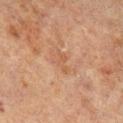Assessment: The lesion was photographed on a routine skin check and not biopsied; there is no pathology result. Background: The total-body-photography lesion software estimated an outline eccentricity of about 0.75 (0 = round, 1 = elongated) and a symmetry-axis asymmetry near 0.45. It also reported roughly 5 lightness units darker than nearby skin and a lesion-to-skin contrast of about 4.5 (normalized; higher = more distinct). The analysis additionally found a border-irregularity rating of about 5/10, a color-variation rating of about 2/10, and a peripheral color-asymmetry measure near 1. And it measured a classifier nevus-likeness of about 0/100 and a detector confidence of about 100 out of 100 that the crop contains a lesion. On the right lower leg. The lesion's longest dimension is about 2.5 mm. A roughly 15 mm field-of-view crop from a total-body skin photograph. This is a cross-polarized tile. A male subject in their 70s.